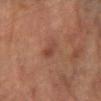Notes:
- notes: total-body-photography surveillance lesion; no biopsy
- acquisition: total-body-photography crop, ~15 mm field of view
- lesion diameter: ≈2.5 mm
- location: the arm
- patient: male, in their mid-80s or older